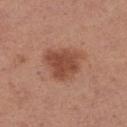notes = catalogued during a skin exam; not biopsied | tile lighting = white-light | anatomic site = the left thigh | acquisition = total-body-photography crop, ~15 mm field of view | patient = female, roughly 30 years of age | diameter = ≈4.5 mm.